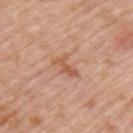Recorded during total-body skin imaging; not selected for excision or biopsy. The patient is a male in their mid-70s. The lesion is located on the left upper arm. An algorithmic analysis of the crop reported a color-variation rating of about 0.5/10 and a peripheral color-asymmetry measure near 0. Imaged with white-light lighting. Measured at roughly 3 mm in maximum diameter. Cropped from a total-body skin-imaging series; the visible field is about 15 mm.